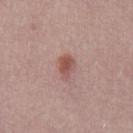Assessment: Part of a total-body skin-imaging series; this lesion was reviewed on a skin check and was not flagged for biopsy. Acquisition and patient details: Cropped from a whole-body photographic skin survey; the tile spans about 15 mm. A male patient, aged 28–32.